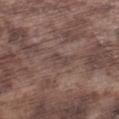Acquisition and patient details: A 15 mm close-up tile from a total-body photography series done for melanoma screening. From the left lower leg. A male subject, aged 73 to 77.